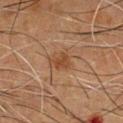The subject is a male aged 63 to 67. A 15 mm crop from a total-body photograph taken for skin-cancer surveillance. The lesion is on the chest. The total-body-photography lesion software estimated a lesion color around L≈37 a*≈19 b*≈30 in CIELAB, roughly 7 lightness units darker than nearby skin, and a normalized lesion–skin contrast near 7. It also reported a border-irregularity rating of about 3.5/10, a within-lesion color-variation index near 1.5/10, and a peripheral color-asymmetry measure near 0.5. About 2.5 mm across. The tile uses cross-polarized illumination.